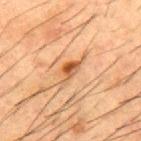{
  "biopsy_status": "not biopsied; imaged during a skin examination",
  "image": {
    "source": "total-body photography crop",
    "field_of_view_mm": 15
  },
  "patient": {
    "sex": "male",
    "age_approx": 50
  },
  "lesion_size": {
    "long_diameter_mm_approx": 4.5
  },
  "site": "back",
  "lighting": "cross-polarized"
}